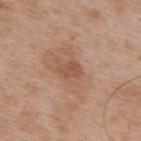Findings:
– follow-up — imaged on a skin check; not biopsied
– imaging modality — total-body-photography crop, ~15 mm field of view
– patient — male, in their 50s
– tile lighting — white-light
– location — the upper back
– diameter — about 2.5 mm